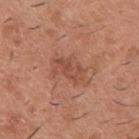Captured during whole-body skin photography for melanoma surveillance; the lesion was not biopsied. A male patient, aged 38–42. Cropped from a whole-body photographic skin survey; the tile spans about 15 mm. Captured under white-light illumination. Automated tile analysis of the lesion measured a border-irregularity index near 5/10 and a within-lesion color-variation index near 3/10. It also reported a nevus-likeness score of about 5/100 and a lesion-detection confidence of about 100/100. The lesion is located on the back.